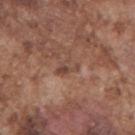Clinical impression:
Part of a total-body skin-imaging series; this lesion was reviewed on a skin check and was not flagged for biopsy.
Context:
A close-up tile cropped from a whole-body skin photograph, about 15 mm across. A male subject about 75 years old. Located on the right upper arm.